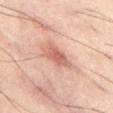Impression: The lesion was photographed on a routine skin check and not biopsied; there is no pathology result. Clinical summary: The lesion is on the abdomen. The total-body-photography lesion software estimated an average lesion color of about L≈58 a*≈24 b*≈26 (CIELAB) and about 11 CIELAB-L* units darker than the surrounding skin. The analysis additionally found a border-irregularity index near 3/10 and a within-lesion color-variation index near 3/10. And it measured a nevus-likeness score of about 65/100 and a detector confidence of about 100 out of 100 that the crop contains a lesion. Cropped from a whole-body photographic skin survey; the tile spans about 15 mm. Longest diameter approximately 4 mm. A male patient, aged 58–62.